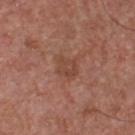* follow-up — catalogued during a skin exam; not biopsied
* size — ~2.5 mm (longest diameter)
* location — the chest
* subject — male, in their mid-50s
* image — ~15 mm crop, total-body skin-cancer survey
* tile lighting — white-light illumination